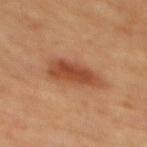follow-up — total-body-photography surveillance lesion; no biopsy | image — ~15 mm crop, total-body skin-cancer survey | image-analysis metrics — a nevus-likeness score of about 95/100 and lesion-presence confidence of about 100/100 | subject — male, in their mid-60s | body site — the mid back | illumination — cross-polarized | lesion diameter — ≈5.5 mm.